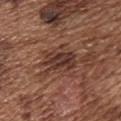This lesion was catalogued during total-body skin photography and was not selected for biopsy. Approximately 4 mm at its widest. Cropped from a whole-body photographic skin survey; the tile spans about 15 mm. Imaged with white-light lighting. The subject is a male approximately 75 years of age. The lesion is located on the chest.